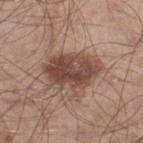workup = total-body-photography surveillance lesion; no biopsy
subject = male, aged 53–57
size = ~6.5 mm (longest diameter)
site = the leg
image source = total-body-photography crop, ~15 mm field of view